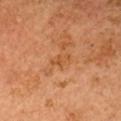Imaged during a routine full-body skin examination; the lesion was not biopsied and no histopathology is available.
Located on the head or neck.
A male patient roughly 60 years of age.
Approximately 2.5 mm at its widest.
A close-up tile cropped from a whole-body skin photograph, about 15 mm across.